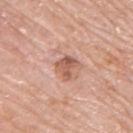Assessment: Imaged during a routine full-body skin examination; the lesion was not biopsied and no histopathology is available. Background: On the upper back. Longest diameter approximately 3 mm. A 15 mm close-up extracted from a 3D total-body photography capture. The total-body-photography lesion software estimated a lesion area of about 5 mm², an outline eccentricity of about 0.5 (0 = round, 1 = elongated), and two-axis asymmetry of about 0.4. The software also gave a mean CIELAB color near L≈57 a*≈24 b*≈30, about 11 CIELAB-L* units darker than the surrounding skin, and a lesion-to-skin contrast of about 7.5 (normalized; higher = more distinct). The software also gave internal color variation of about 3.5 on a 0–10 scale and peripheral color asymmetry of about 1.5. A male subject, aged approximately 70. The tile uses white-light illumination.